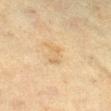| feature | finding |
|---|---|
| patient | female, aged 53 to 57 |
| automated lesion analysis | an area of roughly 3 mm², a shape eccentricity near 0.9, and a symmetry-axis asymmetry near 0.4; a lesion color around L≈55 a*≈13 b*≈33 in CIELAB; a classifier nevus-likeness of about 0/100 and a detector confidence of about 100 out of 100 that the crop contains a lesion |
| diameter | ≈3 mm |
| location | the right lower leg |
| imaging modality | 15 mm crop, total-body photography |
| illumination | cross-polarized illumination |